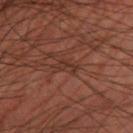Impression:
The lesion was tiled from a total-body skin photograph and was not biopsied.
Image and clinical context:
A male subject, in their mid-60s. Located on the leg. This image is a 15 mm lesion crop taken from a total-body photograph. This is a cross-polarized tile. Measured at roughly 2.5 mm in maximum diameter. An algorithmic analysis of the crop reported an outline eccentricity of about 0.85 (0 = round, 1 = elongated) and two-axis asymmetry of about 0.3.